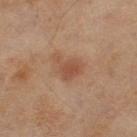follow-up: no biopsy performed (imaged during a skin exam); lesion diameter: ≈3.5 mm; subject: female, roughly 60 years of age; location: the right thigh; image: total-body-photography crop, ~15 mm field of view.